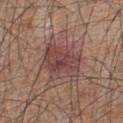The lesion was photographed on a routine skin check and not biopsied; there is no pathology result. Cropped from a total-body skin-imaging series; the visible field is about 15 mm. Longest diameter approximately 5 mm. The subject is a male in their mid- to late 40s. Captured under white-light illumination. Located on the upper back. An algorithmic analysis of the crop reported an outline eccentricity of about 0.65 (0 = round, 1 = elongated) and a symmetry-axis asymmetry near 0.25. The analysis additionally found about 9 CIELAB-L* units darker than the surrounding skin and a lesion-to-skin contrast of about 7.5 (normalized; higher = more distinct). The analysis additionally found an automated nevus-likeness rating near 15 out of 100.